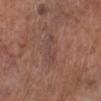follow-up = no biopsy performed (imaged during a skin exam) | image = ~15 mm crop, total-body skin-cancer survey | anatomic site = the left lower leg | subject = male, aged around 70 | lesion diameter = about 3.5 mm.